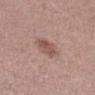notes = catalogued during a skin exam; not biopsied
patient = female, approximately 40 years of age
lighting = white-light
anatomic site = the left lower leg
imaging modality = total-body-photography crop, ~15 mm field of view
automated metrics = a border-irregularity rating of about 2/10 and a peripheral color-asymmetry measure near 1; a detector confidence of about 100 out of 100 that the crop contains a lesion
lesion size = ≈3.5 mm